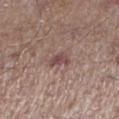Imaged with white-light lighting.
The lesion is located on the left lower leg.
Longest diameter approximately 2.5 mm.
A male subject roughly 65 years of age.
A lesion tile, about 15 mm wide, cut from a 3D total-body photograph.